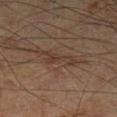Q: Was this lesion biopsied?
A: total-body-photography surveillance lesion; no biopsy
Q: Automated lesion metrics?
A: an area of roughly 5.5 mm², a shape eccentricity near 0.95, and a symmetry-axis asymmetry near 0.5; a mean CIELAB color near L≈35 a*≈16 b*≈23 and a lesion-to-skin contrast of about 6 (normalized; higher = more distinct); a border-irregularity index near 8/10, a color-variation rating of about 0.5/10, and a peripheral color-asymmetry measure near 0; a classifier nevus-likeness of about 0/100 and lesion-presence confidence of about 65/100
Q: Lesion size?
A: ~4.5 mm (longest diameter)
Q: Lesion location?
A: the leg
Q: How was the tile lit?
A: cross-polarized illumination
Q: Patient demographics?
A: male, aged around 70
Q: How was this image acquired?
A: 15 mm crop, total-body photography